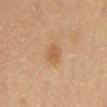No biopsy was performed on this lesion — it was imaged during a full skin examination and was not determined to be concerning.
Captured under cross-polarized illumination.
Longest diameter approximately 2.5 mm.
A roughly 15 mm field-of-view crop from a total-body skin photograph.
A male subject, roughly 60 years of age.